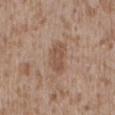Clinical impression:
The lesion was tiled from a total-body skin photograph and was not biopsied.
Acquisition and patient details:
The lesion's longest dimension is about 4 mm. Located on the mid back. A male subject aged approximately 45. A region of skin cropped from a whole-body photographic capture, roughly 15 mm wide.